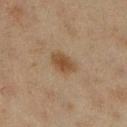A 15 mm crop from a total-body photograph taken for skin-cancer surveillance.
On the right lower leg.
This is a cross-polarized tile.
About 3 mm across.
Automated tile analysis of the lesion measured a nevus-likeness score of about 90/100 and a detector confidence of about 100 out of 100 that the crop contains a lesion.
A female patient, about 40 years old.